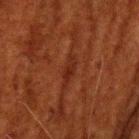Impression: The lesion was tiled from a total-body skin photograph and was not biopsied. Acquisition and patient details: Measured at roughly 3 mm in maximum diameter. Automated tile analysis of the lesion measured an area of roughly 2.5 mm², an eccentricity of roughly 0.9, and two-axis asymmetry of about 0.4. It also reported a lesion color around L≈20 a*≈22 b*≈25 in CIELAB and a normalized lesion–skin contrast near 7. It also reported a border-irregularity index near 4.5/10 and internal color variation of about 0 on a 0–10 scale. The tile uses cross-polarized illumination. Cropped from a whole-body photographic skin survey; the tile spans about 15 mm. From the head or neck. A male patient, roughly 60 years of age.